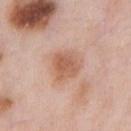Impression: Imaged during a routine full-body skin examination; the lesion was not biopsied and no histopathology is available. Context: This is a white-light tile. Cropped from a whole-body photographic skin survey; the tile spans about 15 mm. The subject is a male in their mid- to late 50s. Located on the chest. The total-body-photography lesion software estimated a classifier nevus-likeness of about 75/100. Measured at roughly 3.5 mm in maximum diameter.